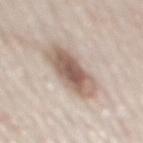biopsy_status: not biopsied; imaged during a skin examination
image:
  source: total-body photography crop
  field_of_view_mm: 15
site: mid back
patient:
  sex: male
  age_approx: 80
lesion_size:
  long_diameter_mm_approx: 6.0